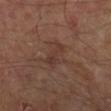Assessment:
No biopsy was performed on this lesion — it was imaged during a full skin examination and was not determined to be concerning.
Background:
A 15 mm close-up tile from a total-body photography series done for melanoma screening. A male patient aged approximately 65. Captured under cross-polarized illumination. On the right lower leg.